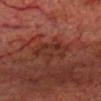Q: Was a biopsy performed?
A: no biopsy performed (imaged during a skin exam)
Q: What is the imaging modality?
A: 15 mm crop, total-body photography
Q: Who is the patient?
A: male, aged 73–77
Q: Lesion location?
A: the head or neck